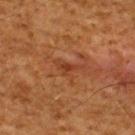Assessment:
Part of a total-body skin-imaging series; this lesion was reviewed on a skin check and was not flagged for biopsy.
Clinical summary:
A male subject roughly 60 years of age. A 15 mm close-up tile from a total-body photography series done for melanoma screening. The total-body-photography lesion software estimated an automated nevus-likeness rating near 0 out of 100 and a lesion-detection confidence of about 100/100. The lesion is located on the upper back. Approximately 3.5 mm at its widest.